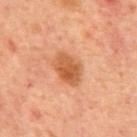No biopsy was performed on this lesion — it was imaged during a full skin examination and was not determined to be concerning. A male patient aged 68–72. A 15 mm crop from a total-body photograph taken for skin-cancer surveillance. The lesion is on the mid back. Imaged with cross-polarized lighting. The lesion-visualizer software estimated about 11 CIELAB-L* units darker than the surrounding skin and a lesion-to-skin contrast of about 8 (normalized; higher = more distinct). It also reported a border-irregularity rating of about 2/10. And it measured an automated nevus-likeness rating near 85 out of 100 and a detector confidence of about 100 out of 100 that the crop contains a lesion.